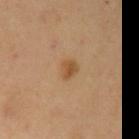TBP lesion metrics = an area of roughly 3 mm², an eccentricity of roughly 0.55, and two-axis asymmetry of about 0.25; a border-irregularity rating of about 2/10, internal color variation of about 2.5 on a 0–10 scale, and peripheral color asymmetry of about 1; a nevus-likeness score of about 90/100 | body site = the left upper arm | tile lighting = cross-polarized illumination | subject = male, aged 63–67 | acquisition = ~15 mm tile from a whole-body skin photo | diameter = ~2 mm (longest diameter).